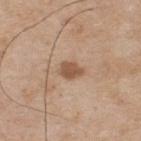Q: Was a biopsy performed?
A: total-body-photography surveillance lesion; no biopsy
Q: How was the tile lit?
A: white-light illumination
Q: Lesion size?
A: ~3 mm (longest diameter)
Q: What kind of image is this?
A: total-body-photography crop, ~15 mm field of view
Q: Who is the patient?
A: male, aged 73–77
Q: Lesion location?
A: the upper back